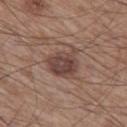<record>
<biopsy_status>not biopsied; imaged during a skin examination</biopsy_status>
<lesion_size>
  <long_diameter_mm_approx>4.0</long_diameter_mm_approx>
</lesion_size>
<automated_metrics>
  <area_mm2_approx>11.0</area_mm2_approx>
  <eccentricity>0.45</eccentricity>
  <shape_asymmetry>0.25</shape_asymmetry>
  <cielab_L>43</cielab_L>
  <cielab_a>18</cielab_a>
  <cielab_b>23</cielab_b>
  <vs_skin_darker_L>10.0</vs_skin_darker_L>
  <vs_skin_contrast_norm>8.5</vs_skin_contrast_norm>
  <border_irregularity_0_10>3.0</border_irregularity_0_10>
  <color_variation_0_10>4.0</color_variation_0_10>
  <peripheral_color_asymmetry>1.0</peripheral_color_asymmetry>
  <lesion_detection_confidence_0_100>100</lesion_detection_confidence_0_100>
</automated_metrics>
<patient>
  <sex>male</sex>
  <age_approx>60</age_approx>
</patient>
<image>
  <source>total-body photography crop</source>
  <field_of_view_mm>15</field_of_view_mm>
</image>
<site>left thigh</site>
</record>